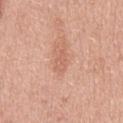| feature | finding |
|---|---|
| notes | imaged on a skin check; not biopsied |
| location | the back |
| image | total-body-photography crop, ~15 mm field of view |
| patient | male, approximately 45 years of age |